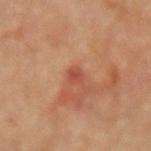Case summary:
- notes · catalogued during a skin exam; not biopsied
- patient · female, approximately 65 years of age
- image · ~15 mm crop, total-body skin-cancer survey
- location · the right forearm